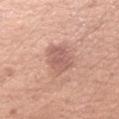Image and clinical context: The lesion is on the left forearm. Cropped from a whole-body photographic skin survey; the tile spans about 15 mm. Captured under white-light illumination. Automated image analysis of the tile measured an average lesion color of about L≈59 a*≈23 b*≈25 (CIELAB), a lesion–skin lightness drop of about 10, and a normalized border contrast of about 6.5. The analysis additionally found a border-irregularity index near 2/10, internal color variation of about 2.5 on a 0–10 scale, and a peripheral color-asymmetry measure near 1. And it measured a classifier nevus-likeness of about 10/100. A female patient in their mid- to late 40s. Longest diameter approximately 4 mm.